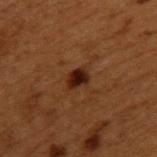Q: Is there a histopathology result?
A: total-body-photography surveillance lesion; no biopsy
Q: How was this image acquired?
A: 15 mm crop, total-body photography
Q: How large is the lesion?
A: ≈2.5 mm
Q: Lesion location?
A: the upper back
Q: What lighting was used for the tile?
A: cross-polarized illumination
Q: Patient demographics?
A: male, approximately 50 years of age